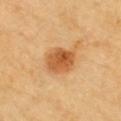Part of a total-body skin-imaging series; this lesion was reviewed on a skin check and was not flagged for biopsy.
The lesion is on the chest.
The total-body-photography lesion software estimated a lesion area of about 9 mm² and a shape-asymmetry score of about 0.15 (0 = symmetric). And it measured a mean CIELAB color near L≈55 a*≈25 b*≈43 and roughly 13 lightness units darker than nearby skin. And it measured a lesion-detection confidence of about 100/100.
A 15 mm crop from a total-body photograph taken for skin-cancer surveillance.
The recorded lesion diameter is about 3.5 mm.
This is a cross-polarized tile.
The subject is a male in their 60s.